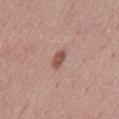The lesion was tiled from a total-body skin photograph and was not biopsied. A male subject aged 43 to 47. Automated image analysis of the tile measured a mean CIELAB color near L≈52 a*≈21 b*≈25, roughly 11 lightness units darker than nearby skin, and a normalized border contrast of about 8. The analysis additionally found an automated nevus-likeness rating near 85 out of 100. From the abdomen. Cropped from a whole-body photographic skin survey; the tile spans about 15 mm.